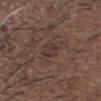No biopsy was performed on this lesion — it was imaged during a full skin examination and was not determined to be concerning. The subject is a male aged around 50. Located on the upper back. Cropped from a whole-body photographic skin survey; the tile spans about 15 mm.